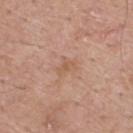Clinical impression: The lesion was photographed on a routine skin check and not biopsied; there is no pathology result.